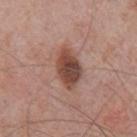Impression:
Recorded during total-body skin imaging; not selected for excision or biopsy.
Acquisition and patient details:
The lesion is located on the chest. A lesion tile, about 15 mm wide, cut from a 3D total-body photograph. An algorithmic analysis of the crop reported a footprint of about 10 mm², an outline eccentricity of about 0.8 (0 = round, 1 = elongated), and a symmetry-axis asymmetry near 0.15. The analysis additionally found an automated nevus-likeness rating near 80 out of 100 and lesion-presence confidence of about 100/100. The subject is a male aged approximately 75. Imaged with white-light lighting. About 5 mm across.